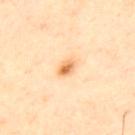Part of a total-body skin-imaging series; this lesion was reviewed on a skin check and was not flagged for biopsy. A male subject, in their mid- to late 40s. The total-body-photography lesion software estimated an eccentricity of roughly 0.75 and two-axis asymmetry of about 0.2. It also reported a within-lesion color-variation index near 6/10 and peripheral color asymmetry of about 2. And it measured a nevus-likeness score of about 90/100 and lesion-presence confidence of about 100/100. About 2.5 mm across. The lesion is on the upper back. A roughly 15 mm field-of-view crop from a total-body skin photograph.